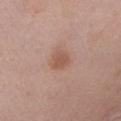Assessment: The lesion was tiled from a total-body skin photograph and was not biopsied. Image and clinical context: On the left upper arm. The tile uses white-light illumination. The subject is a male about 50 years old. The recorded lesion diameter is about 2.5 mm. A roughly 15 mm field-of-view crop from a total-body skin photograph.